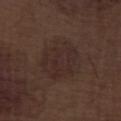notes=total-body-photography surveillance lesion; no biopsy | lighting=white-light | location=the right lower leg | patient=male, aged 68–72 | acquisition=~15 mm crop, total-body skin-cancer survey.